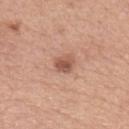| feature | finding |
|---|---|
| biopsy status | imaged on a skin check; not biopsied |
| image | ~15 mm tile from a whole-body skin photo |
| automated metrics | a mean CIELAB color near L≈54 a*≈23 b*≈29 and a lesion–skin lightness drop of about 12; an automated nevus-likeness rating near 70 out of 100 and a lesion-detection confidence of about 100/100 |
| patient | female, aged 58–62 |
| anatomic site | the left upper arm |
| size | about 2.5 mm |